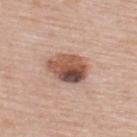Clinical impression: Part of a total-body skin-imaging series; this lesion was reviewed on a skin check and was not flagged for biopsy. Context: On the upper back. The tile uses white-light illumination. A female patient roughly 50 years of age. About 5 mm across. A region of skin cropped from a whole-body photographic capture, roughly 15 mm wide.